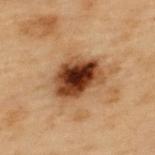Located on the upper back. A male patient aged approximately 55. Cropped from a total-body skin-imaging series; the visible field is about 15 mm. Automated image analysis of the tile measured a classifier nevus-likeness of about 95/100 and a detector confidence of about 100 out of 100 that the crop contains a lesion. This is a cross-polarized tile. About 5.5 mm across.